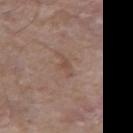No biopsy was performed on this lesion — it was imaged during a full skin examination and was not determined to be concerning. A 15 mm close-up extracted from a 3D total-body photography capture. The patient is a male aged around 80. Located on the left forearm. Automated image analysis of the tile measured a mean CIELAB color near L≈49 a*≈17 b*≈25, a lesion–skin lightness drop of about 6, and a lesion-to-skin contrast of about 5 (normalized; higher = more distinct). This is a white-light tile. Measured at roughly 3 mm in maximum diameter.